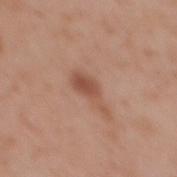The lesion was tiled from a total-body skin photograph and was not biopsied.
A female patient, approximately 35 years of age.
The lesion is on the upper back.
A region of skin cropped from a whole-body photographic capture, roughly 15 mm wide.
The tile uses white-light illumination.
Automated tile analysis of the lesion measured an area of roughly 5.5 mm², an outline eccentricity of about 0.9 (0 = round, 1 = elongated), and a symmetry-axis asymmetry near 0.45. The software also gave a mean CIELAB color near L≈51 a*≈22 b*≈30, a lesion–skin lightness drop of about 10, and a normalized lesion–skin contrast near 7.